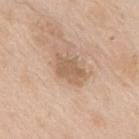Q: Was this lesion biopsied?
A: catalogued during a skin exam; not biopsied
Q: Illumination type?
A: white-light
Q: Automated lesion metrics?
A: an outline eccentricity of about 0.8 (0 = round, 1 = elongated); a mean CIELAB color near L≈60 a*≈16 b*≈32 and a normalized border contrast of about 6.5; a detector confidence of about 100 out of 100 that the crop contains a lesion
Q: What is the lesion's diameter?
A: ~4 mm (longest diameter)
Q: What kind of image is this?
A: total-body-photography crop, ~15 mm field of view
Q: Patient demographics?
A: male, in their mid- to late 60s
Q: Lesion location?
A: the back